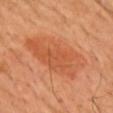{
  "site": "chest",
  "lesion_size": {
    "long_diameter_mm_approx": 8.5
  },
  "automated_metrics": {
    "area_mm2_approx": 30.0,
    "eccentricity": 0.85,
    "border_irregularity_0_10": 2.5,
    "peripheral_color_asymmetry": 1.5
  },
  "image": {
    "source": "total-body photography crop",
    "field_of_view_mm": 15
  },
  "patient": {
    "sex": "male",
    "age_approx": 40
  }
}